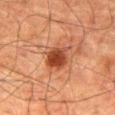Captured during whole-body skin photography for melanoma surveillance; the lesion was not biopsied.
The lesion's longest dimension is about 3.5 mm.
On the right thigh.
This image is a 15 mm lesion crop taken from a total-body photograph.
A male subject aged 78 to 82.
Imaged with cross-polarized lighting.